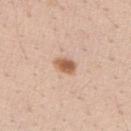Q: Was this lesion biopsied?
A: imaged on a skin check; not biopsied
Q: Where on the body is the lesion?
A: the arm
Q: What are the patient's age and sex?
A: female, aged around 40
Q: How was this image acquired?
A: ~15 mm tile from a whole-body skin photo